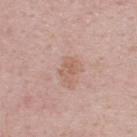The lesion was tiled from a total-body skin photograph and was not biopsied. The tile uses white-light illumination. Approximately 2.5 mm at its widest. A lesion tile, about 15 mm wide, cut from a 3D total-body photograph. Located on the back. The patient is a male aged around 50. The lesion-visualizer software estimated a footprint of about 3.5 mm². The analysis additionally found a border-irregularity index near 4/10 and internal color variation of about 1.5 on a 0–10 scale. And it measured a nevus-likeness score of about 0/100.